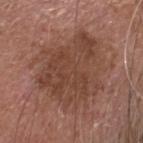follow-up=catalogued during a skin exam; not biopsied
location=the head or neck
image source=15 mm crop, total-body photography
subject=male, about 75 years old
lesion size=~9 mm (longest diameter)
illumination=white-light illumination